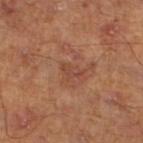Recorded during total-body skin imaging; not selected for excision or biopsy.
The tile uses cross-polarized illumination.
A 15 mm close-up tile from a total-body photography series done for melanoma screening.
Automated image analysis of the tile measured a lesion color around L≈44 a*≈24 b*≈29 in CIELAB and a normalized border contrast of about 5. And it measured a classifier nevus-likeness of about 0/100.
The lesion is located on the left lower leg.
A male patient, in their mid- to late 40s.